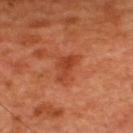biopsy status: no biopsy performed (imaged during a skin exam) | lesion size: ~3.5 mm (longest diameter) | body site: the upper back | acquisition: 15 mm crop, total-body photography | subject: male, approximately 50 years of age.